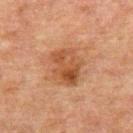Recorded during total-body skin imaging; not selected for excision or biopsy. The recorded lesion diameter is about 4.5 mm. A lesion tile, about 15 mm wide, cut from a 3D total-body photograph. This is a cross-polarized tile. Located on the mid back. A male subject roughly 60 years of age.